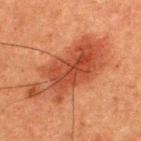Q: Was this lesion biopsied?
A: total-body-photography surveillance lesion; no biopsy
Q: Lesion size?
A: ~10.5 mm (longest diameter)
Q: Lesion location?
A: the upper back
Q: What kind of image is this?
A: ~15 mm tile from a whole-body skin photo
Q: Automated lesion metrics?
A: a lesion–skin lightness drop of about 9
Q: What are the patient's age and sex?
A: male, aged 48 to 52
Q: How was the tile lit?
A: cross-polarized illumination